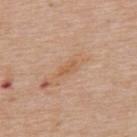Assessment: Recorded during total-body skin imaging; not selected for excision or biopsy. Image and clinical context: Cropped from a whole-body photographic skin survey; the tile spans about 15 mm. Captured under white-light illumination. From the upper back. A male subject, in their mid-60s.